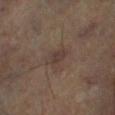Q: Was this lesion biopsied?
A: imaged on a skin check; not biopsied
Q: What are the patient's age and sex?
A: male, aged 63–67
Q: What is the lesion's diameter?
A: ≈2.5 mm
Q: How was the tile lit?
A: cross-polarized illumination
Q: What is the anatomic site?
A: the left lower leg
Q: What is the imaging modality?
A: ~15 mm crop, total-body skin-cancer survey